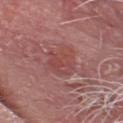notes — imaged on a skin check; not biopsied
body site — the head or neck
tile lighting — white-light illumination
lesion size — ~4.5 mm (longest diameter)
image — ~15 mm crop, total-body skin-cancer survey
patient — male, aged 63 to 67